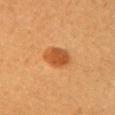notes: no biopsy performed (imaged during a skin exam).